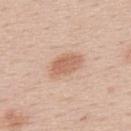The lesion was photographed on a routine skin check and not biopsied; there is no pathology result. Measured at roughly 4.5 mm in maximum diameter. This is a white-light tile. An algorithmic analysis of the crop reported an eccentricity of roughly 0.8 and a shape-asymmetry score of about 0.2 (0 = symmetric). The software also gave an automated nevus-likeness rating near 80 out of 100 and lesion-presence confidence of about 100/100. A region of skin cropped from a whole-body photographic capture, roughly 15 mm wide. The subject is a male in their 60s. From the upper back.